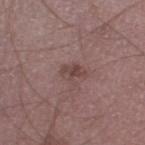biopsy status=imaged on a skin check; not biopsied
image-analysis metrics=a footprint of about 4 mm², a shape eccentricity near 0.8, and two-axis asymmetry of about 0.4; a lesion color around L≈44 a*≈18 b*≈19 in CIELAB, about 8 CIELAB-L* units darker than the surrounding skin, and a normalized lesion–skin contrast near 6.5; a border-irregularity rating of about 3.5/10 and a within-lesion color-variation index near 4/10
anatomic site=the leg
imaging modality=15 mm crop, total-body photography
diameter=about 3 mm
subject=male, in their 60s